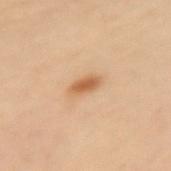workup=imaged on a skin check; not biopsied
patient=female, approximately 50 years of age
location=the left forearm
image source=15 mm crop, total-body photography